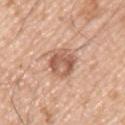The lesion was photographed on a routine skin check and not biopsied; there is no pathology result. Located on the arm. This image is a 15 mm lesion crop taken from a total-body photograph. The recorded lesion diameter is about 4.5 mm. This is a white-light tile. A male subject, aged 53 to 57.